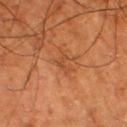Image and clinical context:
A close-up tile cropped from a whole-body skin photograph, about 15 mm across. A male patient aged approximately 80. The lesion is on the left lower leg. Automated tile analysis of the lesion measured a footprint of about 3.5 mm², a shape eccentricity near 0.85, and a shape-asymmetry score of about 0.6 (0 = symmetric). The software also gave a border-irregularity rating of about 6.5/10. Imaged with cross-polarized lighting. Measured at roughly 3 mm in maximum diameter.